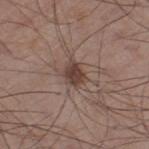Recorded during total-body skin imaging; not selected for excision or biopsy. An algorithmic analysis of the crop reported a lesion area of about 5 mm², an outline eccentricity of about 0.65 (0 = round, 1 = elongated), and a shape-asymmetry score of about 0.25 (0 = symmetric). This image is a 15 mm lesion crop taken from a total-body photograph. The lesion's longest dimension is about 3 mm. From the left thigh. The patient is a male in their 60s. Captured under white-light illumination.